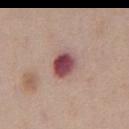<tbp_lesion>
<biopsy_status>not biopsied; imaged during a skin examination</biopsy_status>
<lighting>white-light</lighting>
<image>
  <source>total-body photography crop</source>
  <field_of_view_mm>15</field_of_view_mm>
</image>
<patient>
  <sex>male</sex>
  <age_approx>60</age_approx>
</patient>
<site>abdomen</site>
<lesion_size>
  <long_diameter_mm_approx>3.0</long_diameter_mm_approx>
</lesion_size>
<automated_metrics>
  <cielab_L>47</cielab_L>
  <cielab_a>27</cielab_a>
  <cielab_b>18</cielab_b>
  <vs_skin_darker_L>17.0</vs_skin_darker_L>
  <vs_skin_contrast_norm>12.5</vs_skin_contrast_norm>
  <border_irregularity_0_10>1.0</border_irregularity_0_10>
  <color_variation_0_10>6.0</color_variation_0_10>
  <peripheral_color_asymmetry>2.0</peripheral_color_asymmetry>
  <nevus_likeness_0_100>0</nevus_likeness_0_100>
  <lesion_detection_confidence_0_100>100</lesion_detection_confidence_0_100>
</automated_metrics>
</tbp_lesion>